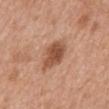| key | value |
|---|---|
| notes | no biopsy performed (imaged during a skin exam) |
| acquisition | total-body-photography crop, ~15 mm field of view |
| automated lesion analysis | an eccentricity of roughly 0.7 and two-axis asymmetry of about 0.25; a lesion color around L≈51 a*≈24 b*≈32 in CIELAB, about 13 CIELAB-L* units darker than the surrounding skin, and a lesion-to-skin contrast of about 8.5 (normalized; higher = more distinct); a border-irregularity rating of about 2.5/10, a within-lesion color-variation index near 3.5/10, and radial color variation of about 1; an automated nevus-likeness rating near 85 out of 100 and lesion-presence confidence of about 100/100 |
| patient | female, about 40 years old |
| body site | the back |
| lesion diameter | ~4 mm (longest diameter) |
| illumination | white-light illumination |